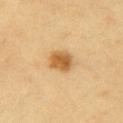Assessment: The lesion was tiled from a total-body skin photograph and was not biopsied. Clinical summary: Captured under cross-polarized illumination. The patient is a male roughly 60 years of age. The lesion is located on the arm. This image is a 15 mm lesion crop taken from a total-body photograph. The lesion-visualizer software estimated a footprint of about 6.5 mm² and a shape eccentricity near 0.5. The analysis additionally found a lesion–skin lightness drop of about 13 and a normalized border contrast of about 9. The software also gave peripheral color asymmetry of about 1. It also reported a classifier nevus-likeness of about 100/100 and a detector confidence of about 100 out of 100 that the crop contains a lesion.